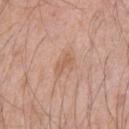{
  "biopsy_status": "not biopsied; imaged during a skin examination",
  "image": {
    "source": "total-body photography crop",
    "field_of_view_mm": 15
  },
  "patient": {
    "sex": "male",
    "age_approx": 45
  },
  "lighting": "white-light",
  "site": "left upper arm",
  "automated_metrics": {
    "eccentricity": 0.85,
    "shape_asymmetry": 0.3,
    "nevus_likeness_0_100": 0,
    "lesion_detection_confidence_0_100": 100
  }
}